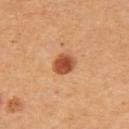Captured under cross-polarized illumination.
The lesion's longest dimension is about 3 mm.
Located on the right upper arm.
The patient is a female about 45 years old.
A 15 mm close-up tile from a total-body photography series done for melanoma screening.